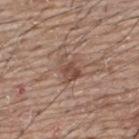– location: the upper back
– tile lighting: white-light illumination
– acquisition: 15 mm crop, total-body photography
– subject: male, aged approximately 65
– TBP lesion metrics: a footprint of about 6 mm² and an outline eccentricity of about 0.65 (0 = round, 1 = elongated); an average lesion color of about L≈48 a*≈18 b*≈26 (CIELAB), roughly 9 lightness units darker than nearby skin, and a normalized lesion–skin contrast near 7; border irregularity of about 3.5 on a 0–10 scale, a color-variation rating of about 3.5/10, and radial color variation of about 1